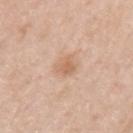This is a white-light tile. The patient is a male aged around 65. Measured at roughly 2.5 mm in maximum diameter. A close-up tile cropped from a whole-body skin photograph, about 15 mm across. On the arm.